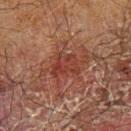follow-up = catalogued during a skin exam; not biopsied
subject = male, about 70 years old
imaging modality = ~15 mm tile from a whole-body skin photo
diameter = ~3.5 mm (longest diameter)
body site = the left forearm